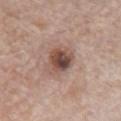Clinical impression: Imaged during a routine full-body skin examination; the lesion was not biopsied and no histopathology is available. Acquisition and patient details: A male subject roughly 85 years of age or older. A close-up tile cropped from a whole-body skin photograph, about 15 mm across. The lesion is located on the chest.